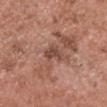Q: Patient demographics?
A: male, about 50 years old
Q: What kind of image is this?
A: total-body-photography crop, ~15 mm field of view
Q: Lesion location?
A: the head or neck
Q: Illumination type?
A: white-light
Q: What is the lesion's diameter?
A: ≈2.5 mm